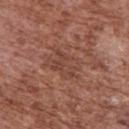Assessment: This lesion was catalogued during total-body skin photography and was not selected for biopsy. Background: Cropped from a total-body skin-imaging series; the visible field is about 15 mm. Captured under white-light illumination. A male patient about 75 years old. The recorded lesion diameter is about 4.5 mm. Located on the upper back.